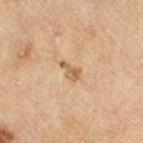biopsy status=imaged on a skin check; not biopsied
image-analysis metrics=an average lesion color of about L≈52 a*≈16 b*≈32 (CIELAB) and a normalized lesion–skin contrast near 7
image source=total-body-photography crop, ~15 mm field of view
subject=female, roughly 55 years of age
body site=the right thigh
lighting=cross-polarized